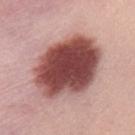workup — total-body-photography surveillance lesion; no biopsy | automated metrics — two-axis asymmetry of about 0.15; border irregularity of about 1.5 on a 0–10 scale, a color-variation rating of about 6.5/10, and peripheral color asymmetry of about 2; an automated nevus-likeness rating near 85 out of 100 and a lesion-detection confidence of about 100/100 | imaging modality — 15 mm crop, total-body photography | subject — male, aged around 30 | illumination — white-light | lesion diameter — ≈8.5 mm.